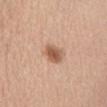The lesion was photographed on a routine skin check and not biopsied; there is no pathology result. From the front of the torso. The tile uses white-light illumination. This image is a 15 mm lesion crop taken from a total-body photograph. A female subject approximately 75 years of age. The total-body-photography lesion software estimated a mean CIELAB color near L≈57 a*≈21 b*≈32, roughly 13 lightness units darker than nearby skin, and a lesion-to-skin contrast of about 8.5 (normalized; higher = more distinct). It also reported a classifier nevus-likeness of about 95/100 and a lesion-detection confidence of about 100/100.